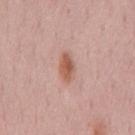notes: total-body-photography surveillance lesion; no biopsy | size: about 3.5 mm | site: the chest | imaging modality: 15 mm crop, total-body photography | patient: male, aged approximately 60 | lighting: white-light.